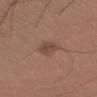<record>
  <biopsy_status>not biopsied; imaged during a skin examination</biopsy_status>
  <image>
    <source>total-body photography crop</source>
    <field_of_view_mm>15</field_of_view_mm>
  </image>
  <site>left upper arm</site>
  <patient>
    <sex>male</sex>
    <age_approx>35</age_approx>
  </patient>
</record>